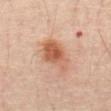Assessment:
Part of a total-body skin-imaging series; this lesion was reviewed on a skin check and was not flagged for biopsy.
Acquisition and patient details:
Located on the abdomen. This is a cross-polarized tile. A 15 mm close-up tile from a total-body photography series done for melanoma screening. A male subject, aged around 55.